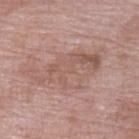Part of a total-body skin-imaging series; this lesion was reviewed on a skin check and was not flagged for biopsy.
A close-up tile cropped from a whole-body skin photograph, about 15 mm across.
On the left lower leg.
About 8.5 mm across.
A female patient aged 68–72.
Automated image analysis of the tile measured an area of roughly 21 mm², an outline eccentricity of about 0.85 (0 = round, 1 = elongated), and two-axis asymmetry of about 0.3. It also reported a lesion color around L≈56 a*≈19 b*≈24 in CIELAB and a normalized lesion–skin contrast near 5. And it measured a border-irregularity rating of about 6/10 and a peripheral color-asymmetry measure near 1.5. The analysis additionally found a nevus-likeness score of about 0/100.